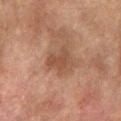Assessment: Part of a total-body skin-imaging series; this lesion was reviewed on a skin check and was not flagged for biopsy. Acquisition and patient details: The tile uses cross-polarized illumination. Measured at roughly 3.5 mm in maximum diameter. A lesion tile, about 15 mm wide, cut from a 3D total-body photograph. Located on the right forearm. The subject is a male roughly 75 years of age.